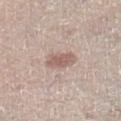workup = catalogued during a skin exam; not biopsied | automated lesion analysis = an average lesion color of about L≈59 a*≈17 b*≈23 (CIELAB) and roughly 10 lightness units darker than nearby skin; a border-irregularity index near 2/10, a within-lesion color-variation index near 2/10, and radial color variation of about 0.5; an automated nevus-likeness rating near 45 out of 100 and a detector confidence of about 100 out of 100 that the crop contains a lesion | image = 15 mm crop, total-body photography | location = the left lower leg | size = about 3.5 mm | subject = male, roughly 70 years of age.